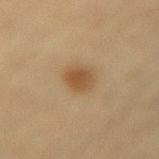This lesion was catalogued during total-body skin photography and was not selected for biopsy. Measured at roughly 3 mm in maximum diameter. The subject is a female roughly 55 years of age. Cropped from a total-body skin-imaging series; the visible field is about 15 mm. The lesion is located on the lower back. Captured under cross-polarized illumination.